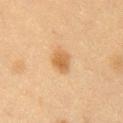Notes:
- follow-up: no biopsy performed (imaged during a skin exam)
- acquisition: ~15 mm crop, total-body skin-cancer survey
- TBP lesion metrics: a lesion area of about 4.5 mm², an eccentricity of roughly 0.65, and a symmetry-axis asymmetry near 0.2; border irregularity of about 2 on a 0–10 scale, internal color variation of about 2.5 on a 0–10 scale, and a peripheral color-asymmetry measure near 0.5; a classifier nevus-likeness of about 95/100 and lesion-presence confidence of about 100/100
- diameter: ~2.5 mm (longest diameter)
- patient: female, aged approximately 40
- anatomic site: the chest
- tile lighting: cross-polarized